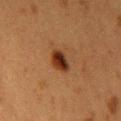Imaged during a routine full-body skin examination; the lesion was not biopsied and no histopathology is available. A female subject in their 40s. The lesion is on the upper back. An algorithmic analysis of the crop reported an average lesion color of about L≈29 a*≈21 b*≈29 (CIELAB), a lesion–skin lightness drop of about 12, and a lesion-to-skin contrast of about 11.5 (normalized; higher = more distinct). And it measured border irregularity of about 2 on a 0–10 scale and peripheral color asymmetry of about 2. Imaged with cross-polarized lighting. Cropped from a whole-body photographic skin survey; the tile spans about 15 mm. Longest diameter approximately 3.5 mm.